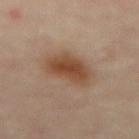Q: Was a biopsy performed?
A: no biopsy performed (imaged during a skin exam)
Q: How was this image acquired?
A: total-body-photography crop, ~15 mm field of view
Q: How was the tile lit?
A: cross-polarized
Q: What is the anatomic site?
A: the abdomen
Q: What are the patient's age and sex?
A: male, in their mid- to late 80s or older
Q: What did automated image analysis measure?
A: an average lesion color of about L≈44 a*≈19 b*≈30 (CIELAB), about 10 CIELAB-L* units darker than the surrounding skin, and a normalized border contrast of about 9; border irregularity of about 3 on a 0–10 scale and radial color variation of about 1